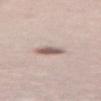| key | value |
|---|---|
| notes | imaged on a skin check; not biopsied |
| imaging modality | total-body-photography crop, ~15 mm field of view |
| location | the leg |
| TBP lesion metrics | an automated nevus-likeness rating near 55 out of 100 and a lesion-detection confidence of about 80/100 |
| patient | male, aged approximately 65 |
| lighting | white-light |